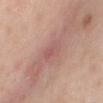• biopsy status · no biopsy performed (imaged during a skin exam)
• diameter · ~3 mm (longest diameter)
• body site · the chest
• image-analysis metrics · an area of roughly 4 mm², an outline eccentricity of about 0.85 (0 = round, 1 = elongated), and a symmetry-axis asymmetry near 0.25; a mean CIELAB color near L≈56 a*≈24 b*≈22 and a lesion–skin lightness drop of about 6; internal color variation of about 2.5 on a 0–10 scale and peripheral color asymmetry of about 1
• subject · female, aged 38–42
• illumination · white-light illumination
• image · 15 mm crop, total-body photography